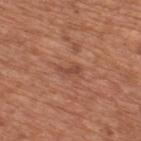* notes: catalogued during a skin exam; not biopsied
* TBP lesion metrics: a border-irregularity index near 6/10, internal color variation of about 0 on a 0–10 scale, and a peripheral color-asymmetry measure near 0
* lesion diameter: about 2.5 mm
* image: ~15 mm tile from a whole-body skin photo
* patient: male, approximately 65 years of age
* illumination: white-light illumination
* anatomic site: the back